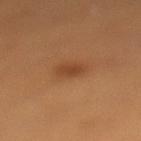Q: Is there a histopathology result?
A: imaged on a skin check; not biopsied
Q: What is the imaging modality?
A: ~15 mm crop, total-body skin-cancer survey
Q: What are the patient's age and sex?
A: female, in their 40s
Q: How large is the lesion?
A: about 2.5 mm
Q: How was the tile lit?
A: cross-polarized
Q: What did automated image analysis measure?
A: an average lesion color of about L≈42 a*≈23 b*≈35 (CIELAB) and a normalized border contrast of about 6.5; a border-irregularity index near 1.5/10, internal color variation of about 1 on a 0–10 scale, and radial color variation of about 0.5; a classifier nevus-likeness of about 90/100 and a detector confidence of about 100 out of 100 that the crop contains a lesion
Q: Where on the body is the lesion?
A: the left lower leg